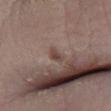{"biopsy_status": "not biopsied; imaged during a skin examination", "lesion_size": {"long_diameter_mm_approx": 1.5}, "site": "right lower leg", "image": {"source": "total-body photography crop", "field_of_view_mm": 15}, "automated_metrics": {"nevus_likeness_0_100": 0}, "lighting": "white-light", "patient": {"sex": "female", "age_approx": 20}}